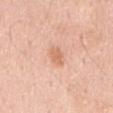Q: Was a biopsy performed?
A: total-body-photography surveillance lesion; no biopsy
Q: Lesion location?
A: the mid back
Q: What kind of image is this?
A: ~15 mm tile from a whole-body skin photo
Q: Patient demographics?
A: male, approximately 60 years of age